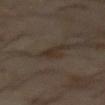Q: Was a biopsy performed?
A: imaged on a skin check; not biopsied
Q: Illumination type?
A: cross-polarized
Q: What kind of image is this?
A: 15 mm crop, total-body photography
Q: Who is the patient?
A: male, aged 58 to 62
Q: Lesion location?
A: the abdomen
Q: Automated lesion metrics?
A: roughly 6 lightness units darker than nearby skin and a normalized border contrast of about 7; a classifier nevus-likeness of about 10/100 and a detector confidence of about 100 out of 100 that the crop contains a lesion
Q: What is the lesion's diameter?
A: ≈3.5 mm